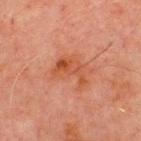Notes:
– follow-up — catalogued during a skin exam; not biopsied
– TBP lesion metrics — an outline eccentricity of about 0.75 (0 = round, 1 = elongated) and a symmetry-axis asymmetry near 0.55
– subject — male, roughly 70 years of age
– anatomic site — the chest
– image source — total-body-photography crop, ~15 mm field of view